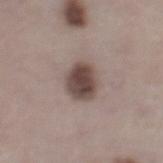Impression:
No biopsy was performed on this lesion — it was imaged during a full skin examination and was not determined to be concerning.
Clinical summary:
From the left lower leg. Approximately 4 mm at its widest. Cropped from a total-body skin-imaging series; the visible field is about 15 mm. A female subject aged around 50. Captured under white-light illumination.